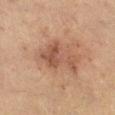{
  "biopsy_status": "not biopsied; imaged during a skin examination",
  "site": "right lower leg",
  "lesion_size": {
    "long_diameter_mm_approx": 5.5
  },
  "image": {
    "source": "total-body photography crop",
    "field_of_view_mm": 15
  },
  "patient": {
    "sex": "female",
    "age_approx": 30
  }
}